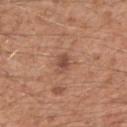workup: imaged on a skin check; not biopsied
image source: ~15 mm crop, total-body skin-cancer survey
lesion size: ~3 mm (longest diameter)
site: the right upper arm
lighting: white-light illumination
patient: male, aged 28–32
TBP lesion metrics: a classifier nevus-likeness of about 50/100 and a detector confidence of about 100 out of 100 that the crop contains a lesion